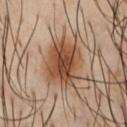Q: Was this lesion biopsied?
A: total-body-photography surveillance lesion; no biopsy
Q: Where on the body is the lesion?
A: the chest
Q: Illumination type?
A: cross-polarized
Q: Who is the patient?
A: male, roughly 40 years of age
Q: What is the imaging modality?
A: ~15 mm crop, total-body skin-cancer survey